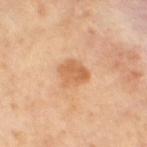Assessment:
Part of a total-body skin-imaging series; this lesion was reviewed on a skin check and was not flagged for biopsy.
Image and clinical context:
This is a cross-polarized tile. Approximately 3 mm at its widest. The subject is a female approximately 65 years of age. Cropped from a whole-body photographic skin survey; the tile spans about 15 mm. Located on the leg.